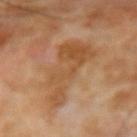This lesion was catalogued during total-body skin photography and was not selected for biopsy.
Located on the right forearm.
Imaged with cross-polarized lighting.
Cropped from a whole-body photographic skin survey; the tile spans about 15 mm.
About 7.5 mm across.
The subject is a male approximately 70 years of age.